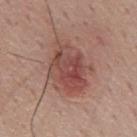The lesion was tiled from a total-body skin photograph and was not biopsied. The lesion is located on the mid back. A region of skin cropped from a whole-body photographic capture, roughly 15 mm wide. Captured under white-light illumination. Automated tile analysis of the lesion measured a border-irregularity rating of about 3/10, a within-lesion color-variation index near 6.5/10, and a peripheral color-asymmetry measure near 2.5. A male subject, aged 58–62. Longest diameter approximately 6.5 mm.